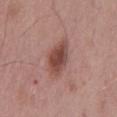No biopsy was performed on this lesion — it was imaged during a full skin examination and was not determined to be concerning. Approximately 5 mm at its widest. Cropped from a total-body skin-imaging series; the visible field is about 15 mm. The lesion is located on the mid back. Automated image analysis of the tile measured an average lesion color of about L≈47 a*≈23 b*≈24 (CIELAB), a lesion–skin lightness drop of about 12, and a normalized border contrast of about 9. A male subject about 55 years old.